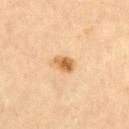Case summary:
• subject — female, aged 58–62
• lighting — cross-polarized
• image source — total-body-photography crop, ~15 mm field of view
• size — ~2.5 mm (longest diameter)
• site — the abdomen